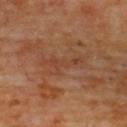Q: Was this lesion biopsied?
A: catalogued during a skin exam; not biopsied
Q: What lighting was used for the tile?
A: cross-polarized
Q: How large is the lesion?
A: about 5 mm
Q: Patient demographics?
A: male, roughly 60 years of age
Q: What is the anatomic site?
A: the upper back
Q: How was this image acquired?
A: total-body-photography crop, ~15 mm field of view
Q: Automated lesion metrics?
A: a color-variation rating of about 0/10 and radial color variation of about 0; a lesion-detection confidence of about 80/100